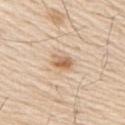follow-up = no biopsy performed (imaged during a skin exam); body site = the right upper arm; diameter = ~2.5 mm (longest diameter); patient = male, roughly 80 years of age; tile lighting = white-light illumination; image-analysis metrics = a border-irregularity index near 2/10, a within-lesion color-variation index near 5/10, and peripheral color asymmetry of about 1.5; image = ~15 mm tile from a whole-body skin photo.